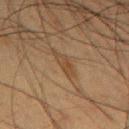Assessment: The lesion was photographed on a routine skin check and not biopsied; there is no pathology result. Background: The lesion is located on the left upper arm. A male patient roughly 55 years of age. The lesion-visualizer software estimated an area of roughly 3.5 mm² and an outline eccentricity of about 0.9 (0 = round, 1 = elongated). The analysis additionally found a color-variation rating of about 2.5/10. A lesion tile, about 15 mm wide, cut from a 3D total-body photograph. The tile uses cross-polarized illumination. The recorded lesion diameter is about 3 mm.